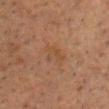Imaged during a routine full-body skin examination; the lesion was not biopsied and no histopathology is available.
On the chest.
The patient is a male in their mid- to late 60s.
The total-body-photography lesion software estimated internal color variation of about 2 on a 0–10 scale and a peripheral color-asymmetry measure near 0.5. It also reported a nevus-likeness score of about 0/100 and lesion-presence confidence of about 100/100.
A 15 mm close-up extracted from a 3D total-body photography capture.